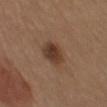Q: What lighting was used for the tile?
A: white-light illumination
Q: Patient demographics?
A: female, in their 40s
Q: Automated lesion metrics?
A: a footprint of about 8.5 mm², a shape eccentricity near 0.6, and a shape-asymmetry score of about 0.2 (0 = symmetric); a lesion color around L≈38 a*≈18 b*≈27 in CIELAB, roughly 10 lightness units darker than nearby skin, and a normalized lesion–skin contrast near 8.5; a border-irregularity index near 2/10, a color-variation rating of about 5/10, and a peripheral color-asymmetry measure near 1.5; a classifier nevus-likeness of about 95/100 and lesion-presence confidence of about 100/100
Q: How large is the lesion?
A: ~3.5 mm (longest diameter)
Q: What kind of image is this?
A: ~15 mm tile from a whole-body skin photo
Q: Lesion location?
A: the front of the torso